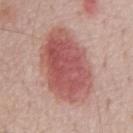Notes:
- biopsy status: imaged on a skin check; not biopsied
- location: the chest
- subject: male, aged approximately 70
- image source: total-body-photography crop, ~15 mm field of view